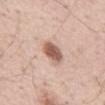No biopsy was performed on this lesion — it was imaged during a full skin examination and was not determined to be concerning. A 15 mm crop from a total-body photograph taken for skin-cancer surveillance. About 3 mm across. The tile uses white-light illumination. A male patient, aged 53 to 57. On the abdomen.